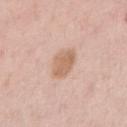Q: Was a biopsy performed?
A: no biopsy performed (imaged during a skin exam)
Q: What kind of image is this?
A: ~15 mm tile from a whole-body skin photo
Q: Patient demographics?
A: male, aged around 50
Q: How large is the lesion?
A: ≈3 mm
Q: What is the anatomic site?
A: the arm
Q: Illumination type?
A: white-light